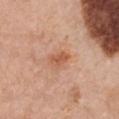Captured under white-light illumination. Measured at roughly 3 mm in maximum diameter. The lesion is on the front of the torso. A 15 mm crop from a total-body photograph taken for skin-cancer surveillance. The subject is a female approximately 60 years of age.